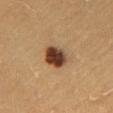| feature | finding |
|---|---|
| follow-up | imaged on a skin check; not biopsied |
| image-analysis metrics | an eccentricity of roughly 0.5 and two-axis asymmetry of about 0.15; a border-irregularity index near 1.5/10 and radial color variation of about 2 |
| anatomic site | the chest |
| subject | female, about 30 years old |
| image source | total-body-photography crop, ~15 mm field of view |
| lighting | cross-polarized |
| diameter | ~3.5 mm (longest diameter) |